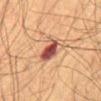Impression: Part of a total-body skin-imaging series; this lesion was reviewed on a skin check and was not flagged for biopsy. Context: This image is a 15 mm lesion crop taken from a total-body photograph. Approximately 4 mm at its widest. From the abdomen. A male patient aged 63–67. This is a cross-polarized tile.